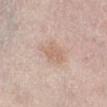Q: Was this lesion biopsied?
A: catalogued during a skin exam; not biopsied
Q: What is the imaging modality?
A: total-body-photography crop, ~15 mm field of view
Q: Who is the patient?
A: female, aged 63–67
Q: Lesion size?
A: about 3 mm
Q: Lesion location?
A: the right lower leg
Q: How was the tile lit?
A: white-light